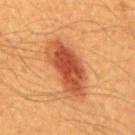<lesion>
  <biopsy_status>not biopsied; imaged during a skin examination</biopsy_status>
  <site>back</site>
  <image>
    <source>total-body photography crop</source>
    <field_of_view_mm>15</field_of_view_mm>
  </image>
  <patient>
    <sex>male</sex>
    <age_approx>60</age_approx>
  </patient>
  <lighting>cross-polarized</lighting>
</lesion>